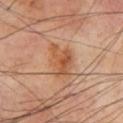Recorded during total-body skin imaging; not selected for excision or biopsy.
Located on the chest.
A male subject roughly 70 years of age.
Measured at roughly 4.5 mm in maximum diameter.
Automated image analysis of the tile measured a shape eccentricity near 0.75 and two-axis asymmetry of about 0.3. And it measured border irregularity of about 3 on a 0–10 scale, a within-lesion color-variation index near 5.5/10, and peripheral color asymmetry of about 1.5. And it measured a classifier nevus-likeness of about 20/100 and lesion-presence confidence of about 100/100.
A 15 mm close-up tile from a total-body photography series done for melanoma screening.
Imaged with cross-polarized lighting.